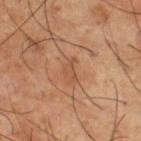Clinical impression:
The lesion was photographed on a routine skin check and not biopsied; there is no pathology result.
Clinical summary:
Cropped from a whole-body photographic skin survey; the tile spans about 15 mm. The lesion is located on the right thigh. About 2.5 mm across. A male patient, aged around 65. Captured under cross-polarized illumination.